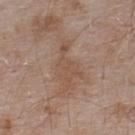Notes:
* tile lighting · white-light
* automated metrics · a lesion area of about 10 mm² and a shape-asymmetry score of about 0.5 (0 = symmetric); a lesion–skin lightness drop of about 6; border irregularity of about 7.5 on a 0–10 scale, a color-variation rating of about 2/10, and radial color variation of about 0.5; a lesion-detection confidence of about 100/100
* image source · total-body-photography crop, ~15 mm field of view
* subject · male, aged around 55
* body site · the upper back
* size · ≈6 mm